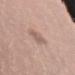Q: Is there a histopathology result?
A: total-body-photography surveillance lesion; no biopsy
Q: What is the imaging modality?
A: ~15 mm tile from a whole-body skin photo
Q: Lesion location?
A: the right thigh
Q: How was the tile lit?
A: white-light
Q: How large is the lesion?
A: about 3 mm
Q: What are the patient's age and sex?
A: female, roughly 25 years of age